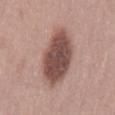Approximately 7 mm at its widest. This image is a 15 mm lesion crop taken from a total-body photograph. This is a white-light tile. A female subject aged around 40. On the lower back. The biopsy diagnosis was a dysplastic (Clark) nevus.